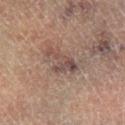follow-up — total-body-photography surveillance lesion; no biopsy | acquisition — ~15 mm crop, total-body skin-cancer survey | patient — male, aged approximately 65 | tile lighting — cross-polarized | anatomic site — the right lower leg | diameter — about 4.5 mm.